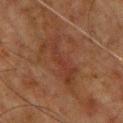<record>
<biopsy_status>not biopsied; imaged during a skin examination</biopsy_status>
<site>chest</site>
<lighting>cross-polarized</lighting>
<lesion_size>
  <long_diameter_mm_approx>7.0</long_diameter_mm_approx>
</lesion_size>
<image>
  <source>total-body photography crop</source>
  <field_of_view_mm>15</field_of_view_mm>
</image>
<automated_metrics>
  <vs_skin_darker_L>5.0</vs_skin_darker_L>
  <vs_skin_contrast_norm>5.0</vs_skin_contrast_norm>
</automated_metrics>
<patient>
  <sex>male</sex>
  <age_approx>60</age_approx>
</patient>
</record>